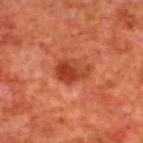No biopsy was performed on this lesion — it was imaged during a full skin examination and was not determined to be concerning.
The patient is a male aged approximately 70.
The lesion's longest dimension is about 3.5 mm.
Imaged with cross-polarized lighting.
On the upper back.
Cropped from a total-body skin-imaging series; the visible field is about 15 mm.